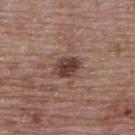The lesion is on the upper back. The subject is a female roughly 65 years of age. A 15 mm close-up extracted from a 3D total-body photography capture.